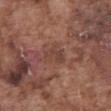<lesion>
  <biopsy_status>not biopsied; imaged during a skin examination</biopsy_status>
  <site>abdomen</site>
  <patient>
    <sex>male</sex>
    <age_approx>75</age_approx>
  </patient>
  <lesion_size>
    <long_diameter_mm_approx>3.0</long_diameter_mm_approx>
  </lesion_size>
  <image>
    <source>total-body photography crop</source>
    <field_of_view_mm>15</field_of_view_mm>
  </image>
</lesion>